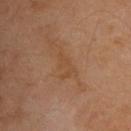workup: total-body-photography surveillance lesion; no biopsy | lesion diameter: ≈4 mm | tile lighting: cross-polarized | imaging modality: ~15 mm tile from a whole-body skin photo | anatomic site: the upper back | automated metrics: a footprint of about 6 mm² and two-axis asymmetry of about 0.5; an average lesion color of about L≈44 a*≈18 b*≈32 (CIELAB), a lesion–skin lightness drop of about 5, and a normalized lesion–skin contrast near 5 | subject: male, aged around 65.